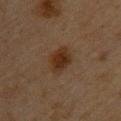– workup: catalogued during a skin exam; not biopsied
– image-analysis metrics: an average lesion color of about L≈26 a*≈16 b*≈26 (CIELAB), roughly 8 lightness units darker than nearby skin, and a lesion-to-skin contrast of about 9.5 (normalized; higher = more distinct); a color-variation rating of about 3.5/10 and a peripheral color-asymmetry measure near 1
– illumination: cross-polarized illumination
– image: ~15 mm tile from a whole-body skin photo
– subject: female, aged 43 to 47
– body site: the upper back
– size: ≈3.5 mm